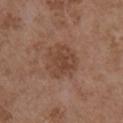* lesion diameter · ≈4.5 mm
* patient · male, roughly 55 years of age
* location · the right upper arm
* tile lighting · white-light illumination
* image source · total-body-photography crop, ~15 mm field of view